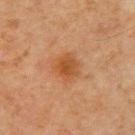No biopsy was performed on this lesion — it was imaged during a full skin examination and was not determined to be concerning. A male subject, about 70 years old. The lesion is on the right upper arm. Captured under cross-polarized illumination. A 15 mm close-up extracted from a 3D total-body photography capture. The recorded lesion diameter is about 3 mm. The lesion-visualizer software estimated a border-irregularity rating of about 2/10, a color-variation rating of about 3/10, and peripheral color asymmetry of about 1.